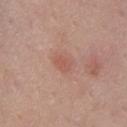The lesion was photographed on a routine skin check and not biopsied; there is no pathology result.
Located on the chest.
A female subject, aged 48 to 52.
About 2.5 mm across.
Captured under white-light illumination.
An algorithmic analysis of the crop reported a footprint of about 3.5 mm², a shape eccentricity near 0.8, and a shape-asymmetry score of about 0.25 (0 = symmetric). It also reported an automated nevus-likeness rating near 25 out of 100 and a detector confidence of about 100 out of 100 that the crop contains a lesion.
Cropped from a whole-body photographic skin survey; the tile spans about 15 mm.